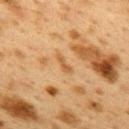workup: total-body-photography surveillance lesion; no biopsy | automated metrics: roughly 7 lightness units darker than nearby skin and a normalized border contrast of about 6; a border-irregularity index near 4.5/10, a color-variation rating of about 0/10, and radial color variation of about 0 | subject: female, aged 38–42 | location: the upper back | acquisition: ~15 mm tile from a whole-body skin photo | illumination: cross-polarized illumination | size: ~3 mm (longest diameter).